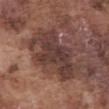<record>
<biopsy_status>not biopsied; imaged during a skin examination</biopsy_status>
<lighting>white-light</lighting>
<site>abdomen</site>
<image>
  <source>total-body photography crop</source>
  <field_of_view_mm>15</field_of_view_mm>
</image>
<lesion_size>
  <long_diameter_mm_approx>7.5</long_diameter_mm_approx>
</lesion_size>
<patient>
  <sex>male</sex>
  <age_approx>75</age_approx>
</patient>
<automated_metrics>
  <area_mm2_approx>27.0</area_mm2_approx>
  <eccentricity>0.75</eccentricity>
  <shape_asymmetry>0.35</shape_asymmetry>
  <cielab_L>38</cielab_L>
  <cielab_a>19</cielab_a>
  <cielab_b>21</cielab_b>
  <vs_skin_contrast_norm>9.0</vs_skin_contrast_norm>
  <border_irregularity_0_10>6.0</border_irregularity_0_10>
  <color_variation_0_10>4.0</color_variation_0_10>
</automated_metrics>
</record>